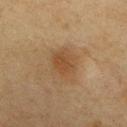Q: Was a biopsy performed?
A: imaged on a skin check; not biopsied